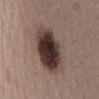  biopsy_status: not biopsied; imaged during a skin examination
  patient:
    sex: female
    age_approx: 45
  site: mid back
  image:
    source: total-body photography crop
    field_of_view_mm: 15